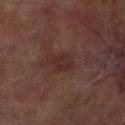Recorded during total-body skin imaging; not selected for excision or biopsy.
A roughly 15 mm field-of-view crop from a total-body skin photograph.
A male patient aged approximately 70.
On the right lower leg.